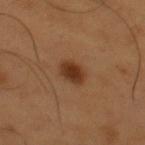Assessment: Part of a total-body skin-imaging series; this lesion was reviewed on a skin check and was not flagged for biopsy. Background: The lesion is on the upper back. A male subject, in their mid- to late 50s. The tile uses cross-polarized illumination. Longest diameter approximately 3 mm. The total-body-photography lesion software estimated a footprint of about 6 mm² and a symmetry-axis asymmetry near 0.15. The analysis additionally found a mean CIELAB color near L≈34 a*≈21 b*≈31. The software also gave a border-irregularity rating of about 1.5/10, a within-lesion color-variation index near 3/10, and a peripheral color-asymmetry measure near 1. The software also gave lesion-presence confidence of about 100/100. A roughly 15 mm field-of-view crop from a total-body skin photograph.